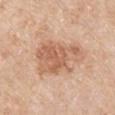The lesion's longest dimension is about 6 mm.
From the left upper arm.
The subject is a male aged 63–67.
A 15 mm close-up extracted from a 3D total-body photography capture.